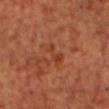The lesion was tiled from a total-body skin photograph and was not biopsied.
Located on the chest.
Cropped from a whole-body photographic skin survey; the tile spans about 15 mm.
A male patient, aged approximately 60.